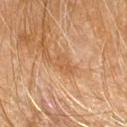{
  "biopsy_status": "not biopsied; imaged during a skin examination",
  "patient": {
    "sex": "male",
    "age_approx": 60
  },
  "site": "right upper arm",
  "lesion_size": {
    "long_diameter_mm_approx": 2.5
  },
  "lighting": "cross-polarized",
  "image": {
    "source": "total-body photography crop",
    "field_of_view_mm": 15
  },
  "automated_metrics": {
    "area_mm2_approx": 3.0,
    "shape_asymmetry": 0.3,
    "nevus_likeness_0_100": 0
  }
}